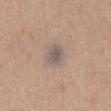Assessment: Captured during whole-body skin photography for melanoma surveillance; the lesion was not biopsied. Image and clinical context: A region of skin cropped from a whole-body photographic capture, roughly 15 mm wide. Longest diameter approximately 2.5 mm. The tile uses white-light illumination. A female patient aged 63–67. From the chest. The lesion-visualizer software estimated a classifier nevus-likeness of about 0/100.